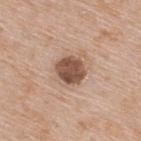Clinical impression:
The lesion was photographed on a routine skin check and not biopsied; there is no pathology result.
Clinical summary:
Captured under white-light illumination. The lesion is on the upper back. A 15 mm close-up extracted from a 3D total-body photography capture. A male patient, about 65 years old. The total-body-photography lesion software estimated a classifier nevus-likeness of about 25/100 and a detector confidence of about 100 out of 100 that the crop contains a lesion. The recorded lesion diameter is about 3.5 mm.